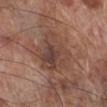This lesion was catalogued during total-body skin photography and was not selected for biopsy. From the right lower leg. Captured under white-light illumination. A male patient, approximately 70 years of age. A 15 mm close-up extracted from a 3D total-body photography capture. The recorded lesion diameter is about 5 mm.